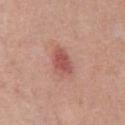{"biopsy_status": "not biopsied; imaged during a skin examination", "site": "chest", "image": {"source": "total-body photography crop", "field_of_view_mm": 15}, "patient": {"sex": "male", "age_approx": 55}}